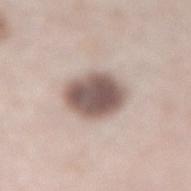Notes:
- notes: catalogued during a skin exam; not biopsied
- automated lesion analysis: a footprint of about 15 mm², an outline eccentricity of about 0.65 (0 = round, 1 = elongated), and a symmetry-axis asymmetry near 0.1; a classifier nevus-likeness of about 35/100
- image: 15 mm crop, total-body photography
- patient: male, in their mid-60s
- illumination: white-light
- anatomic site: the right lower leg
- size: about 5 mm